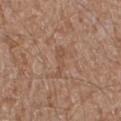Impression:
The lesion was photographed on a routine skin check and not biopsied; there is no pathology result.
Context:
The lesion is located on the leg. A male subject in their mid-60s. Automated image analysis of the tile measured a lesion color around L≈50 a*≈19 b*≈29 in CIELAB and a normalized lesion–skin contrast near 4.5. And it measured border irregularity of about 6 on a 0–10 scale, a color-variation rating of about 0.5/10, and peripheral color asymmetry of about 0. It also reported a lesion-detection confidence of about 100/100. A 15 mm close-up tile from a total-body photography series done for melanoma screening. Longest diameter approximately 4 mm. The tile uses white-light illumination.